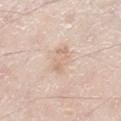{
  "image": {
    "source": "total-body photography crop",
    "field_of_view_mm": 15
  },
  "automated_metrics": {
    "area_mm2_approx": 4.5,
    "nevus_likeness_0_100": 0
  },
  "site": "leg",
  "patient": {
    "sex": "male",
    "age_approx": 60
  }
}